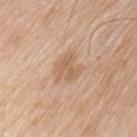biopsy_status: not biopsied; imaged during a skin examination
patient:
  sex: male
  age_approx: 80
lesion_size:
  long_diameter_mm_approx: 3.5
image:
  source: total-body photography crop
  field_of_view_mm: 15
site: chest